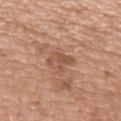Q: Is there a histopathology result?
A: no biopsy performed (imaged during a skin exam)
Q: What are the patient's age and sex?
A: male, aged approximately 50
Q: Illumination type?
A: white-light
Q: How was this image acquired?
A: ~15 mm tile from a whole-body skin photo
Q: Automated lesion metrics?
A: a lesion area of about 5 mm², an outline eccentricity of about 0.8 (0 = round, 1 = elongated), and two-axis asymmetry of about 0.4; an automated nevus-likeness rating near 0 out of 100 and a detector confidence of about 100 out of 100 that the crop contains a lesion
Q: Where on the body is the lesion?
A: the upper back
Q: What is the lesion's diameter?
A: ~3 mm (longest diameter)